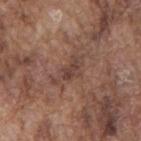| field | value |
|---|---|
| automated metrics | about 7 CIELAB-L* units darker than the surrounding skin and a normalized lesion–skin contrast near 6.5; a border-irregularity rating of about 6/10 and a within-lesion color-variation index near 2.5/10; a classifier nevus-likeness of about 0/100 |
| anatomic site | the mid back |
| acquisition | 15 mm crop, total-body photography |
| lesion diameter | ≈4.5 mm |
| lighting | white-light |
| patient | male, in their mid-70s |